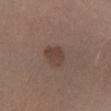Imaged during a routine full-body skin examination; the lesion was not biopsied and no histopathology is available. The lesion's longest dimension is about 3 mm. The tile uses white-light illumination. A 15 mm close-up extracted from a 3D total-body photography capture. The subject is a male approximately 55 years of age. On the leg.